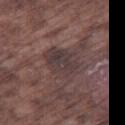| field | value |
|---|---|
| lesion size | ≈5 mm |
| imaging modality | total-body-photography crop, ~15 mm field of view |
| location | the right thigh |
| subject | male, roughly 75 years of age |
| tile lighting | white-light |